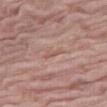Impression:
Imaged during a routine full-body skin examination; the lesion was not biopsied and no histopathology is available.
Clinical summary:
A male subject aged 78 to 82. On the right thigh. A 15 mm close-up tile from a total-body photography series done for melanoma screening. Automated image analysis of the tile measured a lesion area of about 2.5 mm² and an outline eccentricity of about 0.9 (0 = round, 1 = elongated). And it measured a border-irregularity rating of about 4/10 and radial color variation of about 0. The software also gave a classifier nevus-likeness of about 0/100 and lesion-presence confidence of about 60/100. About 2.5 mm across.